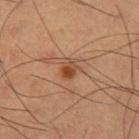Q: Was this lesion biopsied?
A: imaged on a skin check; not biopsied
Q: What are the patient's age and sex?
A: male, in their mid-60s
Q: Lesion location?
A: the right thigh
Q: Automated lesion metrics?
A: a lesion color around L≈45 a*≈23 b*≈33 in CIELAB, about 10 CIELAB-L* units darker than the surrounding skin, and a normalized lesion–skin contrast near 8; lesion-presence confidence of about 100/100
Q: What is the imaging modality?
A: total-body-photography crop, ~15 mm field of view
Q: What is the lesion's diameter?
A: ≈2.5 mm
Q: How was the tile lit?
A: cross-polarized illumination